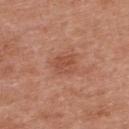| field | value |
|---|---|
| workup | total-body-photography surveillance lesion; no biopsy |
| site | the upper back |
| subject | male, aged 28 to 32 |
| lighting | white-light illumination |
| image-analysis metrics | an area of roughly 4.5 mm², a shape eccentricity near 0.25, and two-axis asymmetry of about 0.25; an average lesion color of about L≈50 a*≈26 b*≈31 (CIELAB); a classifier nevus-likeness of about 20/100 |
| lesion size | ~2.5 mm (longest diameter) |
| image source | ~15 mm crop, total-body skin-cancer survey |